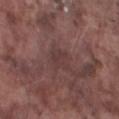Q: Was a biopsy performed?
A: no biopsy performed (imaged during a skin exam)
Q: Where on the body is the lesion?
A: the left lower leg
Q: What did automated image analysis measure?
A: a footprint of about 5.5 mm², an outline eccentricity of about 0.6 (0 = round, 1 = elongated), and a symmetry-axis asymmetry near 0.25; a nevus-likeness score of about 0/100 and lesion-presence confidence of about 75/100
Q: What are the patient's age and sex?
A: male, approximately 75 years of age
Q: What is the imaging modality?
A: 15 mm crop, total-body photography
Q: How large is the lesion?
A: ~3 mm (longest diameter)
Q: What lighting was used for the tile?
A: white-light illumination